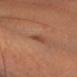The lesion was tiled from a total-body skin photograph and was not biopsied. The lesion is located on the head or neck. Cropped from a whole-body photographic skin survey; the tile spans about 15 mm. A female patient in their mid- to late 30s.